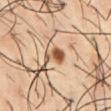Impression:
This lesion was catalogued during total-body skin photography and was not selected for biopsy.
Image and clinical context:
The lesion-visualizer software estimated a lesion area of about 4 mm², an eccentricity of roughly 0.7, and a shape-asymmetry score of about 0.5 (0 = symmetric). Imaged with cross-polarized lighting. On the chest. A 15 mm close-up extracted from a 3D total-body photography capture. A male subject aged around 55.